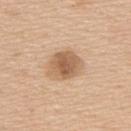Findings:
• workup · catalogued during a skin exam; not biopsied
• diameter · ≈4.5 mm
• lighting · white-light illumination
• acquisition · ~15 mm crop, total-body skin-cancer survey
• patient · male, aged 58–62
• body site · the upper back